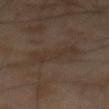| field | value |
|---|---|
| notes | total-body-photography surveillance lesion; no biopsy |
| image | ~15 mm tile from a whole-body skin photo |
| automated lesion analysis | an outline eccentricity of about 0.85 (0 = round, 1 = elongated) and a symmetry-axis asymmetry near 0.3; a mean CIELAB color near L≈31 a*≈12 b*≈21 and a normalized lesion–skin contrast near 4.5 |
| lesion size | ≈4.5 mm |
| tile lighting | cross-polarized |
| subject | male, aged around 65 |